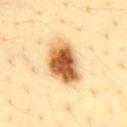{"site": "abdomen", "image": {"source": "total-body photography crop", "field_of_view_mm": 15}, "patient": {"sex": "male", "age_approx": 45}}